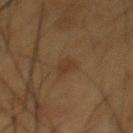No biopsy was performed on this lesion — it was imaged during a full skin examination and was not determined to be concerning.
A male subject aged 53–57.
The lesion's longest dimension is about 3 mm.
A close-up tile cropped from a whole-body skin photograph, about 15 mm across.
The lesion is located on the chest.